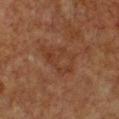Imaged during a routine full-body skin examination; the lesion was not biopsied and no histopathology is available. Measured at roughly 5 mm in maximum diameter. The total-body-photography lesion software estimated a shape eccentricity near 0.8 and two-axis asymmetry of about 0.3. The analysis additionally found a mean CIELAB color near L≈30 a*≈18 b*≈26 and a normalized lesion–skin contrast near 5. And it measured a border-irregularity index near 3.5/10 and a within-lesion color-variation index near 3.5/10. And it measured a classifier nevus-likeness of about 0/100 and a lesion-detection confidence of about 100/100. On the chest. A female patient, approximately 55 years of age. A 15 mm crop from a total-body photograph taken for skin-cancer surveillance.